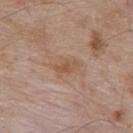Imaged during a routine full-body skin examination; the lesion was not biopsied and no histopathology is available. From the upper back. Captured under white-light illumination. About 3.5 mm across. Automated tile analysis of the lesion measured a lesion area of about 5.5 mm², an outline eccentricity of about 0.8 (0 = round, 1 = elongated), and a symmetry-axis asymmetry near 0.25. And it measured a border-irregularity index near 3/10, a color-variation rating of about 3/10, and peripheral color asymmetry of about 1. A male subject, aged approximately 55. A close-up tile cropped from a whole-body skin photograph, about 15 mm across.